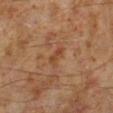| key | value |
|---|---|
| follow-up | total-body-photography surveillance lesion; no biopsy |
| location | the left lower leg |
| image | ~15 mm tile from a whole-body skin photo |
| tile lighting | cross-polarized illumination |
| patient | male, aged around 60 |
| size | ~2.5 mm (longest diameter) |
| automated metrics | a shape eccentricity near 0.85 and a shape-asymmetry score of about 0.3 (0 = symmetric); about 6 CIELAB-L* units darker than the surrounding skin and a lesion-to-skin contrast of about 6 (normalized; higher = more distinct) |